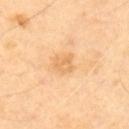Impression: Imaged during a routine full-body skin examination; the lesion was not biopsied and no histopathology is available. Image and clinical context: This is a cross-polarized tile. A roughly 15 mm field-of-view crop from a total-body skin photograph. The lesion is on the upper back. Approximately 2.5 mm at its widest. The subject is a male in their 70s.